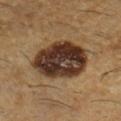Q: Is there a histopathology result?
A: imaged on a skin check; not biopsied
Q: What is the imaging modality?
A: ~15 mm tile from a whole-body skin photo
Q: Lesion size?
A: about 7 mm
Q: What lighting was used for the tile?
A: cross-polarized
Q: Lesion location?
A: the right lower leg
Q: Patient demographics?
A: male, approximately 60 years of age